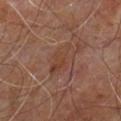  biopsy_status: not biopsied; imaged during a skin examination